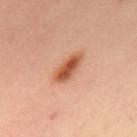Captured during whole-body skin photography for melanoma surveillance; the lesion was not biopsied. This image is a 15 mm lesion crop taken from a total-body photograph. Automated image analysis of the tile measured a footprint of about 7 mm², a shape eccentricity near 0.9, and a shape-asymmetry score of about 0.25 (0 = symmetric). The analysis additionally found an average lesion color of about L≈55 a*≈28 b*≈36 (CIELAB), about 14 CIELAB-L* units darker than the surrounding skin, and a normalized border contrast of about 9.5. The analysis additionally found a border-irregularity rating of about 3/10, internal color variation of about 4 on a 0–10 scale, and radial color variation of about 1. Captured under cross-polarized illumination. From the upper back. The patient is a female roughly 40 years of age.